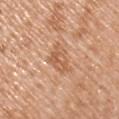Clinical impression:
The lesion was tiled from a total-body skin photograph and was not biopsied.
Clinical summary:
On the left upper arm. A region of skin cropped from a whole-body photographic capture, roughly 15 mm wide. This is a white-light tile. The subject is a male approximately 50 years of age. The lesion-visualizer software estimated a mean CIELAB color near L≈59 a*≈22 b*≈35, a lesion–skin lightness drop of about 9, and a normalized border contrast of about 6. The analysis additionally found border irregularity of about 4.5 on a 0–10 scale, a within-lesion color-variation index near 4.5/10, and radial color variation of about 2. It also reported an automated nevus-likeness rating near 0 out of 100 and a detector confidence of about 100 out of 100 that the crop contains a lesion.